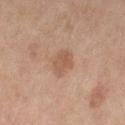Captured during whole-body skin photography for melanoma surveillance; the lesion was not biopsied.
The subject is a female roughly 60 years of age.
The lesion is on the left thigh.
A 15 mm close-up extracted from a 3D total-body photography capture.
About 3 mm across.
The total-body-photography lesion software estimated a shape eccentricity near 0.8 and a shape-asymmetry score of about 0.25 (0 = symmetric). The analysis additionally found a mean CIELAB color near L≈47 a*≈18 b*≈27, roughly 7 lightness units darker than nearby skin, and a lesion-to-skin contrast of about 6 (normalized; higher = more distinct). It also reported a nevus-likeness score of about 15/100 and a lesion-detection confidence of about 100/100.